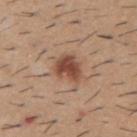<tbp_lesion>
<lesion_size>
  <long_diameter_mm_approx>3.5</long_diameter_mm_approx>
</lesion_size>
<site>upper back</site>
<patient>
  <sex>male</sex>
  <age_approx>60</age_approx>
</patient>
<image>
  <source>total-body photography crop</source>
  <field_of_view_mm>15</field_of_view_mm>
</image>
<lighting>white-light</lighting>
</tbp_lesion>